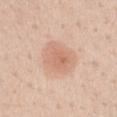Background: Captured under white-light illumination. The lesion's longest dimension is about 4.5 mm. A male subject, aged approximately 60. A region of skin cropped from a whole-body photographic capture, roughly 15 mm wide. On the left upper arm. Automated tile analysis of the lesion measured an automated nevus-likeness rating near 95 out of 100 and a lesion-detection confidence of about 100/100.